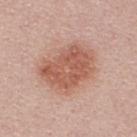{
  "biopsy_status": "not biopsied; imaged during a skin examination"
}